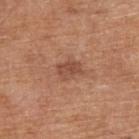Clinical impression: Recorded during total-body skin imaging; not selected for excision or biopsy. Context: The recorded lesion diameter is about 2.5 mm. The subject is a male in their 70s. On the right upper arm. This image is a 15 mm lesion crop taken from a total-body photograph. An algorithmic analysis of the crop reported an area of roughly 4.5 mm², an eccentricity of roughly 0.8, and two-axis asymmetry of about 0.25. And it measured a mean CIELAB color near L≈48 a*≈23 b*≈30 and a lesion-to-skin contrast of about 7 (normalized; higher = more distinct). The software also gave a border-irregularity index near 2.5/10, a within-lesion color-variation index near 2.5/10, and peripheral color asymmetry of about 1. It also reported a nevus-likeness score of about 40/100 and a lesion-detection confidence of about 100/100.